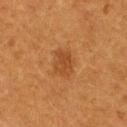No biopsy was performed on this lesion — it was imaged during a full skin examination and was not determined to be concerning.
The subject is a female aged 38 to 42.
Located on the upper back.
A region of skin cropped from a whole-body photographic capture, roughly 15 mm wide.
The total-body-photography lesion software estimated a border-irregularity rating of about 2.5/10 and a peripheral color-asymmetry measure near 0.5.
Longest diameter approximately 3 mm.